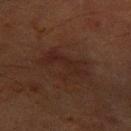  biopsy_status: not biopsied; imaged during a skin examination
  automated_metrics:
    area_mm2_approx: 11.0
    eccentricity: 0.85
    shape_asymmetry: 0.6
    nevus_likeness_0_100: 0
    lesion_detection_confidence_0_100: 100
  patient:
    sex: male
    age_approx: 65
  lighting: cross-polarized
  lesion_size:
    long_diameter_mm_approx: 5.5
  image:
    source: total-body photography crop
    field_of_view_mm: 15
  site: left forearm